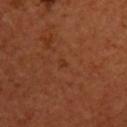* follow-up: no biopsy performed (imaged during a skin exam)
* patient: female, in their 50s
* image: ~15 mm crop, total-body skin-cancer survey
* TBP lesion metrics: a footprint of about 1 mm² and two-axis asymmetry of about 0.4; a within-lesion color-variation index near 0/10 and a peripheral color-asymmetry measure near 0; a nevus-likeness score of about 0/100 and lesion-presence confidence of about 100/100
* body site: the upper back